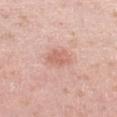Q: Is there a histopathology result?
A: no biopsy performed (imaged during a skin exam)
Q: What is the anatomic site?
A: the left thigh
Q: Lesion size?
A: ~3 mm (longest diameter)
Q: How was this image acquired?
A: ~15 mm tile from a whole-body skin photo
Q: What are the patient's age and sex?
A: female, approximately 40 years of age
Q: Automated lesion metrics?
A: an average lesion color of about L≈64 a*≈24 b*≈28 (CIELAB), about 8 CIELAB-L* units darker than the surrounding skin, and a normalized border contrast of about 5.5; a border-irregularity rating of about 2.5/10 and radial color variation of about 1; an automated nevus-likeness rating near 45 out of 100 and lesion-presence confidence of about 100/100
Q: How was the tile lit?
A: white-light illumination